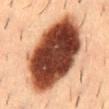• biopsy status · no biopsy performed (imaged during a skin exam)
• image source · ~15 mm tile from a whole-body skin photo
• patient · male, aged approximately 55
• site · the mid back